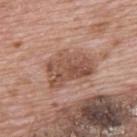Imaged during a routine full-body skin examination; the lesion was not biopsied and no histopathology is available.
This is a white-light tile.
An algorithmic analysis of the crop reported about 11 CIELAB-L* units darker than the surrounding skin and a normalized lesion–skin contrast near 7.5. The software also gave a border-irregularity index near 3.5/10 and a color-variation rating of about 5/10. And it measured a classifier nevus-likeness of about 0/100 and a detector confidence of about 100 out of 100 that the crop contains a lesion.
A 15 mm close-up tile from a total-body photography series done for melanoma screening.
A male subject, in their 70s.
From the upper back.